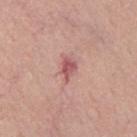workup = imaged on a skin check; not biopsied | subject = male, approximately 55 years of age | image = 15 mm crop, total-body photography | location = the mid back | lesion size = about 3 mm.